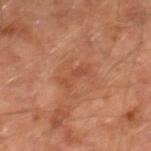The lesion was photographed on a routine skin check and not biopsied; there is no pathology result. Captured under cross-polarized illumination. Cropped from a whole-body photographic skin survey; the tile spans about 15 mm. Located on the right lower leg. A male subject aged around 65. About 3.5 mm across.